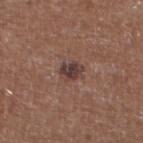Part of a total-body skin-imaging series; this lesion was reviewed on a skin check and was not flagged for biopsy. The lesion's longest dimension is about 2.5 mm. On the left lower leg. A male subject, in their mid-60s. A 15 mm crop from a total-body photograph taken for skin-cancer surveillance. The tile uses white-light illumination.